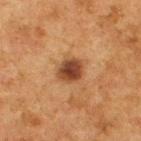No biopsy was performed on this lesion — it was imaged during a full skin examination and was not determined to be concerning. From the upper back. A close-up tile cropped from a whole-body skin photograph, about 15 mm across. A male patient aged 73 to 77.